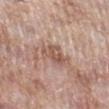* illumination: white-light
* lesion size: ~3.5 mm (longest diameter)
* body site: the leg
* automated lesion analysis: an area of roughly 7 mm²; internal color variation of about 4 on a 0–10 scale and peripheral color asymmetry of about 1.5
* subject: female, aged around 70
* image source: ~15 mm crop, total-body skin-cancer survey